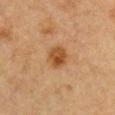Q: Was this lesion biopsied?
A: imaged on a skin check; not biopsied
Q: What is the imaging modality?
A: ~15 mm crop, total-body skin-cancer survey
Q: Who is the patient?
A: female, in their 30s
Q: Where on the body is the lesion?
A: the chest
Q: How large is the lesion?
A: ~2.5 mm (longest diameter)
Q: What lighting was used for the tile?
A: cross-polarized illumination